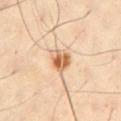* workup — catalogued during a skin exam; not biopsied
* subject — male, in their mid- to late 60s
* body site — the chest
* imaging modality — total-body-photography crop, ~15 mm field of view